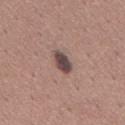Case summary:
- biopsy status: total-body-photography surveillance lesion; no biopsy
- image: 15 mm crop, total-body photography
- diameter: ≈3.5 mm
- patient: male, aged 43–47
- lighting: white-light
- site: the mid back